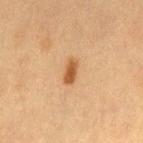Impression:
No biopsy was performed on this lesion — it was imaged during a full skin examination and was not determined to be concerning.
Clinical summary:
The tile uses cross-polarized illumination. Measured at roughly 3 mm in maximum diameter. Cropped from a total-body skin-imaging series; the visible field is about 15 mm. The subject is a female aged approximately 40. Automated tile analysis of the lesion measured an area of roughly 3.5 mm² and a shape eccentricity near 0.85. The analysis additionally found a border-irregularity index near 2.5/10, a within-lesion color-variation index near 2/10, and peripheral color asymmetry of about 0.5. And it measured a classifier nevus-likeness of about 100/100 and a detector confidence of about 100 out of 100 that the crop contains a lesion. From the left thigh.